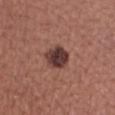{
  "biopsy_status": "not biopsied; imaged during a skin examination",
  "image": {
    "source": "total-body photography crop",
    "field_of_view_mm": 15
  },
  "automated_metrics": {
    "vs_skin_darker_L": 15.0,
    "vs_skin_contrast_norm": 12.5
  },
  "site": "right lower leg",
  "patient": {
    "sex": "female",
    "age_approx": 60
  },
  "lesion_size": {
    "long_diameter_mm_approx": 3.5
  },
  "lighting": "white-light"
}